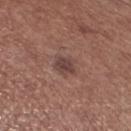Background: An algorithmic analysis of the crop reported an outline eccentricity of about 0.55 (0 = round, 1 = elongated). It also reported a mean CIELAB color near L≈42 a*≈19 b*≈21. The analysis additionally found a border-irregularity index near 2.5/10, a color-variation rating of about 3/10, and peripheral color asymmetry of about 1. The analysis additionally found a lesion-detection confidence of about 100/100. The lesion is on the left lower leg. This is a white-light tile. A roughly 15 mm field-of-view crop from a total-body skin photograph. The patient is a male aged approximately 70.